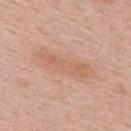{
  "biopsy_status": "not biopsied; imaged during a skin examination",
  "image": {
    "source": "total-body photography crop",
    "field_of_view_mm": 15
  },
  "lighting": "white-light",
  "patient": {
    "sex": "male",
    "age_approx": 55
  },
  "lesion_size": {
    "long_diameter_mm_approx": 7.0
  },
  "site": "back"
}